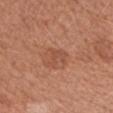Assessment: The lesion was photographed on a routine skin check and not biopsied; there is no pathology result. Background: A female subject aged 53–57. Automated tile analysis of the lesion measured a footprint of about 3 mm², an outline eccentricity of about 0.75 (0 = round, 1 = elongated), and a symmetry-axis asymmetry near 0.6. It also reported an average lesion color of about L≈50 a*≈25 b*≈32 (CIELAB), a lesion–skin lightness drop of about 6, and a lesion-to-skin contrast of about 4.5 (normalized; higher = more distinct). It also reported a border-irregularity index near 6/10 and a color-variation rating of about 0/10. And it measured lesion-presence confidence of about 100/100. A lesion tile, about 15 mm wide, cut from a 3D total-body photograph. The tile uses white-light illumination. The lesion is on the left forearm. About 2.5 mm across.